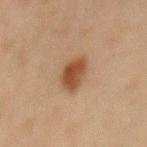Assessment:
The lesion was tiled from a total-body skin photograph and was not biopsied.
Acquisition and patient details:
About 3.5 mm across. Imaged with cross-polarized lighting. On the mid back. An algorithmic analysis of the crop reported a mean CIELAB color near L≈42 a*≈19 b*≈30. The software also gave a lesion-detection confidence of about 100/100. A male patient aged approximately 65. Cropped from a whole-body photographic skin survey; the tile spans about 15 mm.